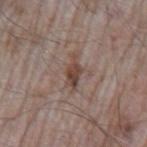From the left upper arm. About 4 mm across. A 15 mm close-up extracted from a 3D total-body photography capture. A male patient aged around 65.